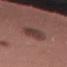Clinical impression:
Imaged during a routine full-body skin examination; the lesion was not biopsied and no histopathology is available.
Context:
The lesion is on the left thigh. Automated image analysis of the tile measured roughly 10 lightness units darker than nearby skin and a lesion-to-skin contrast of about 8 (normalized; higher = more distinct). It also reported a border-irregularity index near 3.5/10 and radial color variation of about 1.5. This is a white-light tile. A region of skin cropped from a whole-body photographic capture, roughly 15 mm wide. About 5 mm across. A female subject about 50 years old.